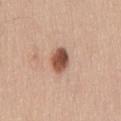No biopsy was performed on this lesion — it was imaged during a full skin examination and was not determined to be concerning. An algorithmic analysis of the crop reported a lesion area of about 6 mm² and an outline eccentricity of about 0.75 (0 = round, 1 = elongated). The software also gave a border-irregularity rating of about 1.5/10, internal color variation of about 5 on a 0–10 scale, and radial color variation of about 2. Captured under white-light illumination. A male subject aged 58 to 62. A 15 mm close-up tile from a total-body photography series done for melanoma screening. On the mid back.